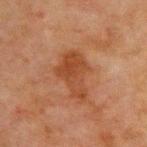<case>
  <biopsy_status>not biopsied; imaged during a skin examination</biopsy_status>
  <lesion_size>
    <long_diameter_mm_approx>5.5</long_diameter_mm_approx>
  </lesion_size>
  <lighting>cross-polarized</lighting>
  <site>chest</site>
  <image>
    <source>total-body photography crop</source>
    <field_of_view_mm>15</field_of_view_mm>
  </image>
  <patient>
    <sex>male</sex>
    <age_approx>65</age_approx>
  </patient>
</case>